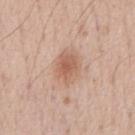Q: Was this lesion biopsied?
A: imaged on a skin check; not biopsied
Q: What lighting was used for the tile?
A: white-light
Q: Lesion size?
A: ≈4.5 mm
Q: How was this image acquired?
A: 15 mm crop, total-body photography
Q: Who is the patient?
A: male, aged around 55
Q: Where on the body is the lesion?
A: the chest